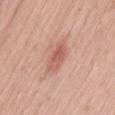Findings:
* follow-up · imaged on a skin check; not biopsied
* imaging modality · ~15 mm tile from a whole-body skin photo
* subject · female, aged approximately 55
* location · the lower back
* illumination · white-light